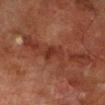Captured during whole-body skin photography for melanoma surveillance; the lesion was not biopsied. About 3 mm across. A male subject, aged around 70. The lesion is on the leg. Imaged with cross-polarized lighting. Cropped from a total-body skin-imaging series; the visible field is about 15 mm.